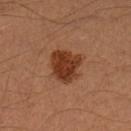Captured during whole-body skin photography for melanoma surveillance; the lesion was not biopsied. Imaged with cross-polarized lighting. On the left lower leg. This image is a 15 mm lesion crop taken from a total-body photograph. A male patient, approximately 40 years of age. Approximately 4 mm at its widest.